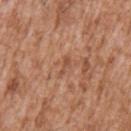Case summary:
- follow-up — no biopsy performed (imaged during a skin exam)
- image source — total-body-photography crop, ~15 mm field of view
- TBP lesion metrics — a lesion area of about 2 mm² and an eccentricity of roughly 0.95; a border-irregularity rating of about 6.5/10, internal color variation of about 0 on a 0–10 scale, and peripheral color asymmetry of about 0; a classifier nevus-likeness of about 0/100 and lesion-presence confidence of about 55/100
- anatomic site — the left upper arm
- illumination — white-light illumination
- lesion diameter — about 2.5 mm
- subject — male, aged approximately 45